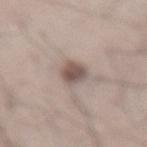No biopsy was performed on this lesion — it was imaged during a full skin examination and was not determined to be concerning.